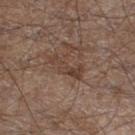Assessment: Part of a total-body skin-imaging series; this lesion was reviewed on a skin check and was not flagged for biopsy.